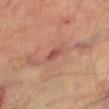Part of a total-body skin-imaging series; this lesion was reviewed on a skin check and was not flagged for biopsy. Cropped from a whole-body photographic skin survey; the tile spans about 15 mm. On the right thigh. Measured at roughly 2.5 mm in maximum diameter. Imaged with cross-polarized lighting. A male patient, aged around 70.